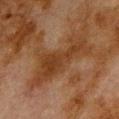Clinical impression: The lesion was photographed on a routine skin check and not biopsied; there is no pathology result. Acquisition and patient details: The lesion is located on the upper back. A roughly 15 mm field-of-view crop from a total-body skin photograph. A male patient aged around 80.